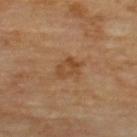Assessment: Imaged during a routine full-body skin examination; the lesion was not biopsied and no histopathology is available. Context: The patient is a male aged approximately 70. A 15 mm close-up tile from a total-body photography series done for melanoma screening. On the upper back. The lesion's longest dimension is about 3.5 mm. Automated image analysis of the tile measured an average lesion color of about L≈45 a*≈20 b*≈35 (CIELAB), a lesion–skin lightness drop of about 7, and a normalized lesion–skin contrast near 6.5.